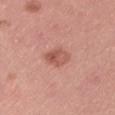Recorded during total-body skin imaging; not selected for excision or biopsy. The patient is a male aged 38–42. Located on the lower back. Cropped from a total-body skin-imaging series; the visible field is about 15 mm. The lesion's longest dimension is about 3 mm.